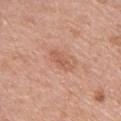Imaged during a routine full-body skin examination; the lesion was not biopsied and no histopathology is available. A close-up tile cropped from a whole-body skin photograph, about 15 mm across. A female patient, aged 58–62. The lesion's longest dimension is about 3.5 mm. From the left upper arm.